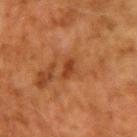Imaged during a routine full-body skin examination; the lesion was not biopsied and no histopathology is available.
A male patient about 70 years old.
On the right upper arm.
An algorithmic analysis of the crop reported an outline eccentricity of about 0.85 (0 = round, 1 = elongated) and two-axis asymmetry of about 0.25. It also reported internal color variation of about 1.5 on a 0–10 scale and a peripheral color-asymmetry measure near 0.5.
Imaged with cross-polarized lighting.
Cropped from a total-body skin-imaging series; the visible field is about 15 mm.
About 2.5 mm across.